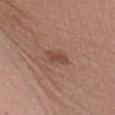<record>
<biopsy_status>not biopsied; imaged during a skin examination</biopsy_status>
<lighting>white-light</lighting>
<image>
  <source>total-body photography crop</source>
  <field_of_view_mm>15</field_of_view_mm>
</image>
<site>left upper arm</site>
<lesion_size>
  <long_diameter_mm_approx>3.0</long_diameter_mm_approx>
</lesion_size>
<patient>
  <sex>female</sex>
  <age_approx>45</age_approx>
</patient>
</record>